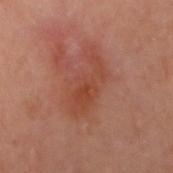Q: Was this lesion biopsied?
A: total-body-photography surveillance lesion; no biopsy
Q: What are the patient's age and sex?
A: female, roughly 60 years of age
Q: Illumination type?
A: cross-polarized
Q: What is the imaging modality?
A: 15 mm crop, total-body photography
Q: What is the lesion's diameter?
A: about 6.5 mm
Q: What is the anatomic site?
A: the left arm
Q: What did automated image analysis measure?
A: a lesion area of about 13 mm² and a shape eccentricity near 0.9; an automated nevus-likeness rating near 5 out of 100 and a lesion-detection confidence of about 100/100